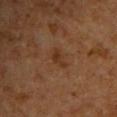{"biopsy_status": "not biopsied; imaged during a skin examination", "image": {"source": "total-body photography crop", "field_of_view_mm": 15}, "lesion_size": {"long_diameter_mm_approx": 3.0}, "patient": {"sex": "male", "age_approx": 60}, "site": "chest"}